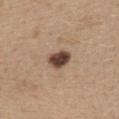A lesion tile, about 15 mm wide, cut from a 3D total-body photograph.
An algorithmic analysis of the crop reported an outline eccentricity of about 0.7 (0 = round, 1 = elongated) and a shape-asymmetry score of about 0.2 (0 = symmetric). It also reported an average lesion color of about L≈44 a*≈17 b*≈25 (CIELAB), about 18 CIELAB-L* units darker than the surrounding skin, and a normalized lesion–skin contrast near 13.5. It also reported border irregularity of about 2 on a 0–10 scale and a peripheral color-asymmetry measure near 1.
The tile uses white-light illumination.
The subject is a female aged approximately 45.
Measured at roughly 3.5 mm in maximum diameter.
From the chest.